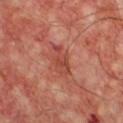Recorded during total-body skin imaging; not selected for excision or biopsy.
Located on the chest.
A male patient, aged approximately 55.
The tile uses cross-polarized illumination.
A roughly 15 mm field-of-view crop from a total-body skin photograph.